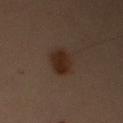Assessment:
Recorded during total-body skin imaging; not selected for excision or biopsy.
Clinical summary:
Imaged with cross-polarized lighting. From the right upper arm. A roughly 15 mm field-of-view crop from a total-body skin photograph. The lesion's longest dimension is about 3 mm. A male patient, in their mid-50s.